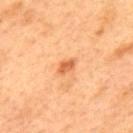The lesion was tiled from a total-body skin photograph and was not biopsied. The recorded lesion diameter is about 2.5 mm. A male subject, aged 48–52. Imaged with cross-polarized lighting. The lesion is on the upper back. Automated tile analysis of the lesion measured a mean CIELAB color near L≈64 a*≈30 b*≈44 and a normalized border contrast of about 7.5. And it measured a nevus-likeness score of about 50/100 and a lesion-detection confidence of about 100/100. A roughly 15 mm field-of-view crop from a total-body skin photograph.